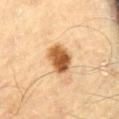Clinical impression:
Recorded during total-body skin imaging; not selected for excision or biopsy.
Context:
The tile uses cross-polarized illumination. On the abdomen. A 15 mm close-up extracted from a 3D total-body photography capture. A male patient, roughly 70 years of age. Measured at roughly 3.5 mm in maximum diameter.